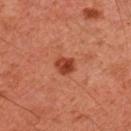<case>
<site>right upper arm</site>
<image>
  <source>total-body photography crop</source>
  <field_of_view_mm>15</field_of_view_mm>
</image>
<patient>
  <sex>male</sex>
  <age_approx>45</age_approx>
</patient>
<lesion_size>
  <long_diameter_mm_approx>2.5</long_diameter_mm_approx>
</lesion_size>
<automated_metrics>
  <vs_skin_darker_L>11.0</vs_skin_darker_L>
  <vs_skin_contrast_norm>9.0</vs_skin_contrast_norm>
  <border_irregularity_0_10>2.0</border_irregularity_0_10>
  <color_variation_0_10>4.0</color_variation_0_10>
  <peripheral_color_asymmetry>1.5</peripheral_color_asymmetry>
  <lesion_detection_confidence_0_100>100</lesion_detection_confidence_0_100>
</automated_metrics>
</case>